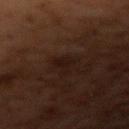Q: Was this lesion biopsied?
A: catalogued during a skin exam; not biopsied
Q: Patient demographics?
A: male, aged around 60
Q: Where on the body is the lesion?
A: the right upper arm
Q: How was the tile lit?
A: cross-polarized
Q: How large is the lesion?
A: ≈2.5 mm
Q: What is the imaging modality?
A: total-body-photography crop, ~15 mm field of view